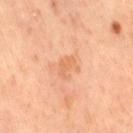Case summary:
* patient: female, aged 63 to 67
* diameter: about 3 mm
* imaging modality: total-body-photography crop, ~15 mm field of view
* TBP lesion metrics: a footprint of about 3 mm² and two-axis asymmetry of about 0.4; a lesion–skin lightness drop of about 7 and a normalized lesion–skin contrast near 5.5; a nevus-likeness score of about 0/100 and a detector confidence of about 100 out of 100 that the crop contains a lesion
* location: the right thigh
* illumination: cross-polarized